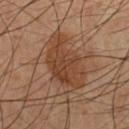Assessment:
No biopsy was performed on this lesion — it was imaged during a full skin examination and was not determined to be concerning.
Image and clinical context:
Approximately 7 mm at its widest. Automated image analysis of the tile measured an area of roughly 20 mm² and two-axis asymmetry of about 0.35. And it measured a lesion color around L≈40 a*≈20 b*≈30 in CIELAB, a lesion–skin lightness drop of about 9, and a lesion-to-skin contrast of about 8 (normalized; higher = more distinct). And it measured border irregularity of about 4.5 on a 0–10 scale, a within-lesion color-variation index near 4/10, and a peripheral color-asymmetry measure near 1.5. On the chest. The patient is a male roughly 60 years of age. Cropped from a whole-body photographic skin survey; the tile spans about 15 mm.